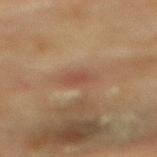notes: catalogued during a skin exam; not biopsied
subject: female, roughly 80 years of age
automated lesion analysis: an outline eccentricity of about 0.85 (0 = round, 1 = elongated) and two-axis asymmetry of about 0.2; a classifier nevus-likeness of about 0/100 and lesion-presence confidence of about 100/100
illumination: cross-polarized
image: 15 mm crop, total-body photography
anatomic site: the mid back
lesion size: about 3.5 mm